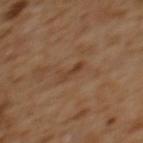workup: no biopsy performed (imaged during a skin exam); location: the upper back; image source: 15 mm crop, total-body photography; patient: female, aged approximately 55; diameter: ≈3 mm; lighting: cross-polarized.